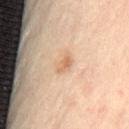Imaged during a routine full-body skin examination; the lesion was not biopsied and no histopathology is available.
A 15 mm crop from a total-body photograph taken for skin-cancer surveillance.
Imaged with cross-polarized lighting.
From the back.
The subject is a male aged around 65.
The lesion's longest dimension is about 2.5 mm.
Automated tile analysis of the lesion measured a footprint of about 3 mm², an outline eccentricity of about 0.85 (0 = round, 1 = elongated), and a symmetry-axis asymmetry near 0.35. The analysis additionally found a border-irregularity index near 3/10 and a within-lesion color-variation index near 2/10. It also reported an automated nevus-likeness rating near 55 out of 100 and a lesion-detection confidence of about 100/100.